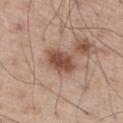Imaged during a routine full-body skin examination; the lesion was not biopsied and no histopathology is available. About 4 mm across. The lesion-visualizer software estimated an area of roughly 7.5 mm², a shape eccentricity near 0.8, and a shape-asymmetry score of about 0.25 (0 = symmetric). The software also gave a lesion-to-skin contrast of about 10 (normalized; higher = more distinct). A roughly 15 mm field-of-view crop from a total-body skin photograph. On the right thigh. A male patient aged 58 to 62.